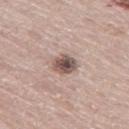<case>
  <site>left thigh</site>
  <image>
    <source>total-body photography crop</source>
    <field_of_view_mm>15</field_of_view_mm>
  </image>
  <lesion_size>
    <long_diameter_mm_approx>3.0</long_diameter_mm_approx>
  </lesion_size>
  <patient>
    <sex>male</sex>
    <age_approx>65</age_approx>
  </patient>
</case>